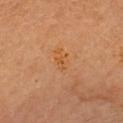diameter: ≈3 mm | subject: female, roughly 60 years of age | acquisition: total-body-photography crop, ~15 mm field of view | anatomic site: the head or neck | tile lighting: cross-polarized illumination.